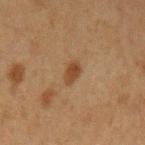Q: Is there a histopathology result?
A: catalogued during a skin exam; not biopsied
Q: Patient demographics?
A: male, aged 48–52
Q: What kind of image is this?
A: total-body-photography crop, ~15 mm field of view
Q: Lesion location?
A: the left upper arm